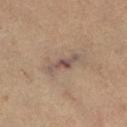The lesion was tiled from a total-body skin photograph and was not biopsied.